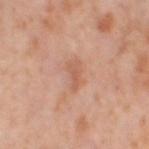Findings:
- biopsy status: catalogued during a skin exam; not biopsied
- patient: female, aged 53 to 57
- tile lighting: cross-polarized illumination
- imaging modality: total-body-photography crop, ~15 mm field of view
- site: the leg
- lesion diameter: about 3 mm
- automated metrics: a lesion area of about 4 mm² and two-axis asymmetry of about 0.4; a classifier nevus-likeness of about 0/100